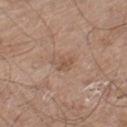Q: Is there a histopathology result?
A: no biopsy performed (imaged during a skin exam)